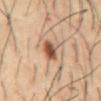illumination: cross-polarized | diameter: about 3 mm | subject: male, about 55 years old | site: the chest | acquisition: total-body-photography crop, ~15 mm field of view.